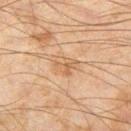The total-body-photography lesion software estimated an outline eccentricity of about 0.65 (0 = round, 1 = elongated). Located on the left thigh. A male patient, about 45 years old. This image is a 15 mm lesion crop taken from a total-body photograph. The lesion's longest dimension is about 3 mm. Captured under cross-polarized illumination.